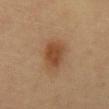Captured under cross-polarized illumination.
A 15 mm crop from a total-body photograph taken for skin-cancer surveillance.
From the front of the torso.
About 4 mm across.
Automated tile analysis of the lesion measured a lesion area of about 9.5 mm², an eccentricity of roughly 0.7, and a symmetry-axis asymmetry near 0.25.
The patient is a female about 60 years old.